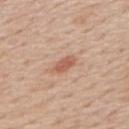Context: A male patient, about 60 years old. The tile uses white-light illumination. Automated image analysis of the tile measured a lesion color around L≈58 a*≈23 b*≈31 in CIELAB and a normalized lesion–skin contrast near 7. The software also gave border irregularity of about 2.5 on a 0–10 scale, a within-lesion color-variation index near 2/10, and peripheral color asymmetry of about 1. It also reported an automated nevus-likeness rating near 55 out of 100. A 15 mm close-up tile from a total-body photography series done for melanoma screening. The lesion is located on the upper back. The recorded lesion diameter is about 3 mm.